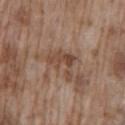A male subject, aged 73–77.
The lesion is on the mid back.
A region of skin cropped from a whole-body photographic capture, roughly 15 mm wide.